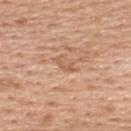<tbp_lesion>
  <lighting>white-light</lighting>
  <image>
    <source>total-body photography crop</source>
    <field_of_view_mm>15</field_of_view_mm>
  </image>
  <automated_metrics>
    <area_mm2_approx>2.0</area_mm2_approx>
    <eccentricity>0.9</eccentricity>
    <shape_asymmetry>0.45</shape_asymmetry>
    <border_irregularity_0_10>5.0</border_irregularity_0_10>
    <color_variation_0_10>0.0</color_variation_0_10>
    <peripheral_color_asymmetry>0.0</peripheral_color_asymmetry>
    <nevus_likeness_0_100>0</nevus_likeness_0_100>
    <lesion_detection_confidence_0_100>100</lesion_detection_confidence_0_100>
  </automated_metrics>
  <patient>
    <sex>female</sex>
    <age_approx>55</age_approx>
  </patient>
  <site>upper back</site>
</tbp_lesion>